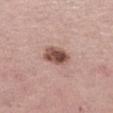follow-up — no biopsy performed (imaged during a skin exam) | site — the left thigh | TBP lesion metrics — a lesion area of about 7 mm² and an eccentricity of roughly 0.55; a color-variation rating of about 6/10 and a peripheral color-asymmetry measure near 2 | image source — ~15 mm crop, total-body skin-cancer survey | subject — female, approximately 45 years of age.